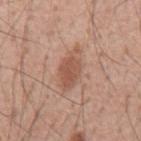Part of a total-body skin-imaging series; this lesion was reviewed on a skin check and was not flagged for biopsy. The total-body-photography lesion software estimated a lesion area of about 9 mm², a shape eccentricity near 0.85, and a shape-asymmetry score of about 0.15 (0 = symmetric). The lesion's longest dimension is about 5 mm. Cropped from a whole-body photographic skin survey; the tile spans about 15 mm. Imaged with white-light lighting. A male patient, in their mid-50s. The lesion is on the mid back.